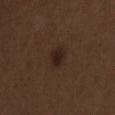The lesion was photographed on a routine skin check and not biopsied; there is no pathology result. A roughly 15 mm field-of-view crop from a total-body skin photograph. A male patient in their 70s. On the chest.